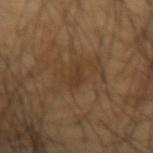body site: the left forearm
subject: male, aged 63 to 67
automated metrics: a footprint of about 3.5 mm² and an eccentricity of roughly 0.9; a mean CIELAB color near L≈36 a*≈15 b*≈30, about 5 CIELAB-L* units darker than the surrounding skin, and a lesion-to-skin contrast of about 5 (normalized; higher = more distinct); a border-irregularity rating of about 3/10, a within-lesion color-variation index near 1/10, and a peripheral color-asymmetry measure near 0
acquisition: ~15 mm tile from a whole-body skin photo
lesion size: ~3 mm (longest diameter)